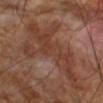Assessment: Part of a total-body skin-imaging series; this lesion was reviewed on a skin check and was not flagged for biopsy. Background: The tile uses cross-polarized illumination. A 15 mm close-up extracted from a 3D total-body photography capture. On the arm. The subject is a male aged around 70.